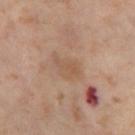Assessment: No biopsy was performed on this lesion — it was imaged during a full skin examination and was not determined to be concerning. Context: A 15 mm close-up tile from a total-body photography series done for melanoma screening. Located on the left thigh. The total-body-photography lesion software estimated a symmetry-axis asymmetry near 0.25. The analysis additionally found a mean CIELAB color near L≈56 a*≈17 b*≈31 and a lesion-to-skin contrast of about 4.5 (normalized; higher = more distinct). And it measured a color-variation rating of about 3.5/10. Measured at roughly 4 mm in maximum diameter. A female subject in their mid- to late 50s.